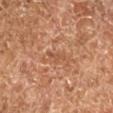Captured during whole-body skin photography for melanoma surveillance; the lesion was not biopsied. A 15 mm close-up extracted from a 3D total-body photography capture. Located on the left lower leg. The patient is approximately 65 years of age. The recorded lesion diameter is about 3 mm.